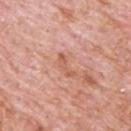workup=imaged on a skin check; not biopsied
anatomic site=the upper back
subject=male, about 80 years old
image source=15 mm crop, total-body photography
diameter=≈3 mm
tile lighting=white-light illumination
automated lesion analysis=a lesion color around L≈59 a*≈26 b*≈31 in CIELAB and roughly 8 lightness units darker than nearby skin; border irregularity of about 5.5 on a 0–10 scale, internal color variation of about 0 on a 0–10 scale, and a peripheral color-asymmetry measure near 0; a detector confidence of about 100 out of 100 that the crop contains a lesion